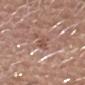The lesion was tiled from a total-body skin photograph and was not biopsied. A male patient roughly 80 years of age. On the chest. Measured at roughly 3.5 mm in maximum diameter. A 15 mm close-up extracted from a 3D total-body photography capture. The lesion-visualizer software estimated a footprint of about 3.5 mm², a shape eccentricity near 0.95, and a shape-asymmetry score of about 0.25 (0 = symmetric). The software also gave an average lesion color of about L≈52 a*≈21 b*≈27 (CIELAB) and a normalized border contrast of about 6. The software also gave a border-irregularity index near 3.5/10, a color-variation rating of about 0.5/10, and peripheral color asymmetry of about 0. The analysis additionally found an automated nevus-likeness rating near 0 out of 100. This is a white-light tile.